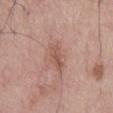Case summary:
• notes: no biopsy performed (imaged during a skin exam)
• site: the mid back
• tile lighting: white-light
• acquisition: ~15 mm tile from a whole-body skin photo
• size: about 4 mm
• subject: male, aged 53 to 57
• automated lesion analysis: a footprint of about 5 mm², a shape eccentricity near 0.9, and a shape-asymmetry score of about 0.25 (0 = symmetric); internal color variation of about 2 on a 0–10 scale and a peripheral color-asymmetry measure near 0.5